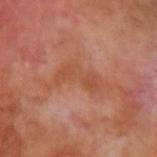Recorded during total-body skin imaging; not selected for excision or biopsy. Automated tile analysis of the lesion measured a lesion-to-skin contrast of about 5.5 (normalized; higher = more distinct). A close-up tile cropped from a whole-body skin photograph, about 15 mm across. The recorded lesion diameter is about 7 mm. This is a cross-polarized tile. The lesion is on the left upper arm. The subject is a male aged approximately 70.